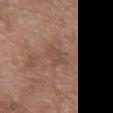follow-up = imaged on a skin check; not biopsied | anatomic site = the chest | size = about 3.5 mm | lighting = white-light illumination | patient = female, aged 68 to 72 | acquisition = ~15 mm tile from a whole-body skin photo | automated metrics = a shape eccentricity near 0.9 and two-axis asymmetry of about 0.3; an average lesion color of about L≈48 a*≈19 b*≈26 (CIELAB), about 6 CIELAB-L* units darker than the surrounding skin, and a normalized lesion–skin contrast near 4.5.